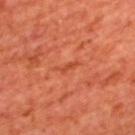Part of a total-body skin-imaging series; this lesion was reviewed on a skin check and was not flagged for biopsy.
On the upper back.
An algorithmic analysis of the crop reported an area of roughly 2 mm², an outline eccentricity of about 0.95 (0 = round, 1 = elongated), and a shape-asymmetry score of about 0.6 (0 = symmetric). The analysis additionally found an average lesion color of about L≈44 a*≈32 b*≈37 (CIELAB) and about 6 CIELAB-L* units darker than the surrounding skin. It also reported a border-irregularity index near 6.5/10 and a within-lesion color-variation index near 0/10. The analysis additionally found a classifier nevus-likeness of about 0/100 and a lesion-detection confidence of about 70/100.
Measured at roughly 3 mm in maximum diameter.
A lesion tile, about 15 mm wide, cut from a 3D total-body photograph.
A male subject aged 63–67.
This is a cross-polarized tile.